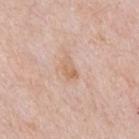biopsy status = catalogued during a skin exam; not biopsied
diameter = ≈2.5 mm
illumination = white-light illumination
anatomic site = the chest
patient = male, roughly 50 years of age
acquisition = 15 mm crop, total-body photography